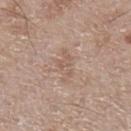  biopsy_status: not biopsied; imaged during a skin examination
  site: left lower leg
  patient:
    sex: male
    age_approx: 65
  image:
    source: total-body photography crop
    field_of_view_mm: 15